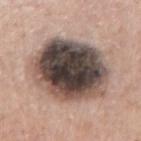Q: Is there a histopathology result?
A: total-body-photography surveillance lesion; no biopsy
Q: What lighting was used for the tile?
A: white-light illumination
Q: What are the patient's age and sex?
A: male, aged 73 to 77
Q: Lesion location?
A: the right upper arm
Q: What is the imaging modality?
A: ~15 mm tile from a whole-body skin photo